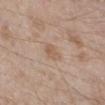Imaged during a routine full-body skin examination; the lesion was not biopsied and no histopathology is available. About 3 mm across. This image is a 15 mm lesion crop taken from a total-body photograph. From the leg. A male patient, aged 43 to 47.